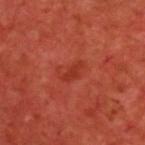This lesion was catalogued during total-body skin photography and was not selected for biopsy. The lesion is on the back. Imaged with cross-polarized lighting. Longest diameter approximately 2.5 mm. A region of skin cropped from a whole-body photographic capture, roughly 15 mm wide. The subject is a male aged approximately 60. The lesion-visualizer software estimated a nevus-likeness score of about 0/100 and a detector confidence of about 100 out of 100 that the crop contains a lesion.